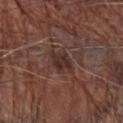The tile uses white-light illumination.
About 3.5 mm across.
The lesion is on the right forearm.
The subject is a male in their 70s.
Cropped from a total-body skin-imaging series; the visible field is about 15 mm.
The total-body-photography lesion software estimated an automated nevus-likeness rating near 5 out of 100 and a detector confidence of about 95 out of 100 that the crop contains a lesion.